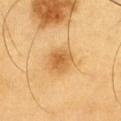Q: Was this lesion biopsied?
A: catalogued during a skin exam; not biopsied
Q: How was the tile lit?
A: cross-polarized illumination
Q: Who is the patient?
A: male, approximately 60 years of age
Q: What is the anatomic site?
A: the chest
Q: What kind of image is this?
A: total-body-photography crop, ~15 mm field of view
Q: Automated lesion metrics?
A: a footprint of about 7 mm², an eccentricity of roughly 0.2, and two-axis asymmetry of about 0.2; internal color variation of about 3.5 on a 0–10 scale and peripheral color asymmetry of about 1; an automated nevus-likeness rating near 95 out of 100 and lesion-presence confidence of about 100/100